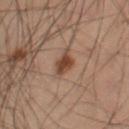notes: no biopsy performed (imaged during a skin exam); lighting: cross-polarized illumination; anatomic site: the leg; imaging modality: total-body-photography crop, ~15 mm field of view; subject: male, aged around 55.